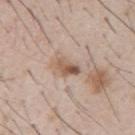{"biopsy_status": "not biopsied; imaged during a skin examination", "lighting": "white-light", "patient": {"sex": "male", "age_approx": 55}, "image": {"source": "total-body photography crop", "field_of_view_mm": 15}}